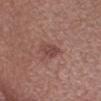Clinical impression:
The lesion was photographed on a routine skin check and not biopsied; there is no pathology result.
Background:
This is a white-light tile. The lesion-visualizer software estimated a footprint of about 4 mm² and a symmetry-axis asymmetry near 0.3. It also reported a mean CIELAB color near L≈44 a*≈22 b*≈23 and a normalized lesion–skin contrast near 6.5. The subject is a male aged 43–47. Cropped from a total-body skin-imaging series; the visible field is about 15 mm. The lesion's longest dimension is about 3 mm. On the head or neck.